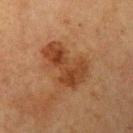<tbp_lesion>
<biopsy_status>not biopsied; imaged during a skin examination</biopsy_status>
<patient>
  <sex>female</sex>
  <age_approx>55</age_approx>
</patient>
<site>left upper arm</site>
<image>
  <source>total-body photography crop</source>
  <field_of_view_mm>15</field_of_view_mm>
</image>
<lighting>cross-polarized</lighting>
<lesion_size>
  <long_diameter_mm_approx>6.0</long_diameter_mm_approx>
</lesion_size>
<automated_metrics>
  <eccentricity>0.8</eccentricity>
  <shape_asymmetry>0.45</shape_asymmetry>
  <border_irregularity_0_10>5.5</border_irregularity_0_10>
  <color_variation_0_10>4.0</color_variation_0_10>
  <nevus_likeness_0_100>5</nevus_likeness_0_100>
  <lesion_detection_confidence_0_100>100</lesion_detection_confidence_0_100>
</automated_metrics>
</tbp_lesion>